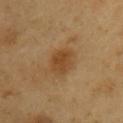Assessment:
Captured during whole-body skin photography for melanoma surveillance; the lesion was not biopsied.
Background:
Located on the arm. A close-up tile cropped from a whole-body skin photograph, about 15 mm across. The patient is a male about 60 years old.